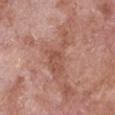Impression: Recorded during total-body skin imaging; not selected for excision or biopsy. Image and clinical context: A 15 mm close-up tile from a total-body photography series done for melanoma screening. A female subject approximately 75 years of age. This is a white-light tile. The lesion is located on the chest. Longest diameter approximately 6.5 mm. The lesion-visualizer software estimated a footprint of about 9.5 mm² and a shape-asymmetry score of about 0.6 (0 = symmetric). The software also gave border irregularity of about 8 on a 0–10 scale.